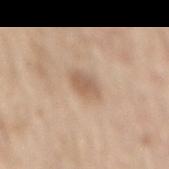biopsy status=imaged on a skin check; not biopsied
patient=male, approximately 70 years of age
anatomic site=the mid back
acquisition=total-body-photography crop, ~15 mm field of view